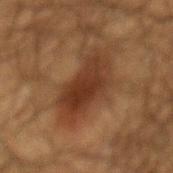Part of a total-body skin-imaging series; this lesion was reviewed on a skin check and was not flagged for biopsy. The lesion-visualizer software estimated a classifier nevus-likeness of about 35/100 and a lesion-detection confidence of about 100/100. Approximately 7 mm at its widest. A region of skin cropped from a whole-body photographic capture, roughly 15 mm wide. Captured under cross-polarized illumination. From the back. A male patient aged around 65.